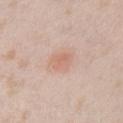{"biopsy_status": "not biopsied; imaged during a skin examination", "image": {"source": "total-body photography crop", "field_of_view_mm": 15}, "patient": {"sex": "female", "age_approx": 25}, "lesion_size": {"long_diameter_mm_approx": 3.0}, "site": "chest", "lighting": "white-light"}